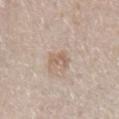Recorded during total-body skin imaging; not selected for excision or biopsy. The subject is a female aged 28–32. The lesion-visualizer software estimated a nevus-likeness score of about 0/100 and lesion-presence confidence of about 100/100. The lesion is located on the left forearm. A close-up tile cropped from a whole-body skin photograph, about 15 mm across. This is a white-light tile. Longest diameter approximately 3 mm.